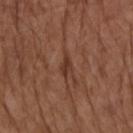Assessment: Imaged during a routine full-body skin examination; the lesion was not biopsied and no histopathology is available. Acquisition and patient details: A male patient, aged approximately 75. The tile uses white-light illumination. Located on the left upper arm. The lesion's longest dimension is about 2.5 mm. A 15 mm close-up extracted from a 3D total-body photography capture.